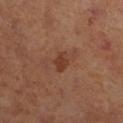Clinical impression: Captured during whole-body skin photography for melanoma surveillance; the lesion was not biopsied. Image and clinical context: A close-up tile cropped from a whole-body skin photograph, about 15 mm across. Located on the right lower leg. Automated image analysis of the tile measured an average lesion color of about L≈39 a*≈23 b*≈30 (CIELAB), about 8 CIELAB-L* units darker than the surrounding skin, and a lesion-to-skin contrast of about 7 (normalized; higher = more distinct). Approximately 2.5 mm at its widest. The tile uses cross-polarized illumination. The subject is female.